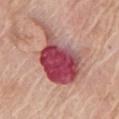biopsy status: no biopsy performed (imaged during a skin exam); image source: 15 mm crop, total-body photography; lesion diameter: about 9 mm; tile lighting: white-light; location: the chest; patient: male, about 65 years old.